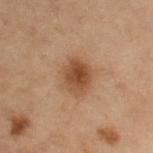Q: Was a biopsy performed?
A: no biopsy performed (imaged during a skin exam)
Q: What is the lesion's diameter?
A: ~4 mm (longest diameter)
Q: What are the patient's age and sex?
A: female, roughly 55 years of age
Q: Lesion location?
A: the left arm
Q: How was the tile lit?
A: cross-polarized
Q: What is the imaging modality?
A: total-body-photography crop, ~15 mm field of view